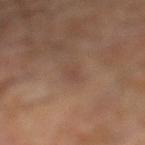notes=no biopsy performed (imaged during a skin exam)
location=the leg
automated lesion analysis=an eccentricity of roughly 0.9; a mean CIELAB color near L≈46 a*≈18 b*≈27, about 6 CIELAB-L* units darker than the surrounding skin, and a normalized lesion–skin contrast near 4.5; a border-irregularity index near 3.5/10, a color-variation rating of about 0/10, and a peripheral color-asymmetry measure near 0
size=~3 mm (longest diameter)
image=~15 mm tile from a whole-body skin photo
tile lighting=cross-polarized illumination